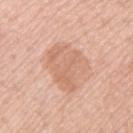biopsy_status: not biopsied; imaged during a skin examination
image:
  source: total-body photography crop
  field_of_view_mm: 15
patient:
  sex: female
  age_approx: 75
site: left thigh
lighting: white-light